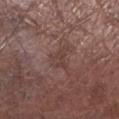Q: Was a biopsy performed?
A: catalogued during a skin exam; not biopsied
Q: Lesion size?
A: about 2.5 mm
Q: Lesion location?
A: the leg
Q: How was this image acquired?
A: total-body-photography crop, ~15 mm field of view
Q: What lighting was used for the tile?
A: white-light
Q: Who is the patient?
A: male, approximately 55 years of age